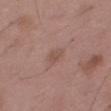Notes:
* workup · no biopsy performed (imaged during a skin exam)
* patient · male, about 50 years old
* size · ~2.5 mm (longest diameter)
* location · the right thigh
* imaging modality · ~15 mm crop, total-body skin-cancer survey
* tile lighting · white-light illumination